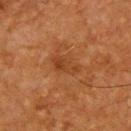A male subject, in their mid- to late 60s. The recorded lesion diameter is about 3.5 mm. The lesion is located on the upper back. A region of skin cropped from a whole-body photographic capture, roughly 15 mm wide.